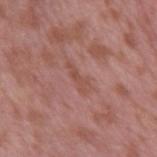Clinical impression: This lesion was catalogued during total-body skin photography and was not selected for biopsy. Image and clinical context: The lesion is on the right upper arm. The tile uses white-light illumination. Longest diameter approximately 3.5 mm. The subject is a male in their 40s. A 15 mm close-up tile from a total-body photography series done for melanoma screening.